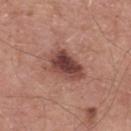<record>
<biopsy_status>not biopsied; imaged during a skin examination</biopsy_status>
<lighting>white-light</lighting>
<patient>
  <sex>male</sex>
  <age_approx>55</age_approx>
</patient>
<site>mid back</site>
<image>
  <source>total-body photography crop</source>
  <field_of_view_mm>15</field_of_view_mm>
</image>
<automated_metrics>
  <area_mm2_approx>13.0</area_mm2_approx>
  <shape_asymmetry>0.3</shape_asymmetry>
  <nevus_likeness_0_100>70</nevus_likeness_0_100>
  <lesion_detection_confidence_0_100>100</lesion_detection_confidence_0_100>
</automated_metrics>
<lesion_size>
  <long_diameter_mm_approx>5.0</long_diameter_mm_approx>
</lesion_size>
</record>